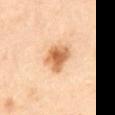Q: Is there a histopathology result?
A: imaged on a skin check; not biopsied
Q: What did automated image analysis measure?
A: an average lesion color of about L≈66 a*≈23 b*≈40 (CIELAB)
Q: How was this image acquired?
A: total-body-photography crop, ~15 mm field of view
Q: Lesion size?
A: ~4 mm (longest diameter)
Q: Lesion location?
A: the mid back
Q: What are the patient's age and sex?
A: female, aged 58 to 62
Q: What lighting was used for the tile?
A: cross-polarized illumination